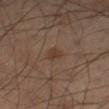This lesion was catalogued during total-body skin photography and was not selected for biopsy.
A male patient, roughly 50 years of age.
From the left leg.
The lesion's longest dimension is about 2.5 mm.
A lesion tile, about 15 mm wide, cut from a 3D total-body photograph.